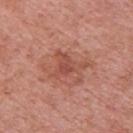workup: total-body-photography surveillance lesion; no biopsy | patient: male, aged 58 to 62 | acquisition: total-body-photography crop, ~15 mm field of view | tile lighting: white-light illumination | body site: the upper back.